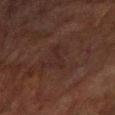Part of a total-body skin-imaging series; this lesion was reviewed on a skin check and was not flagged for biopsy. A male patient, in their mid-60s. Cropped from a total-body skin-imaging series; the visible field is about 15 mm. The recorded lesion diameter is about 3.5 mm. The tile uses cross-polarized illumination. The lesion is located on the right forearm. The lesion-visualizer software estimated an eccentricity of roughly 0.8 and a shape-asymmetry score of about 0.5 (0 = symmetric). The analysis additionally found a nevus-likeness score of about 0/100 and a lesion-detection confidence of about 95/100.